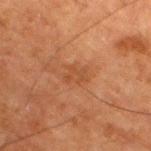Findings:
– imaging modality: total-body-photography crop, ~15 mm field of view
– site: the right thigh
– illumination: cross-polarized illumination
– diameter: ~2.5 mm (longest diameter)
– patient: male, in their 80s